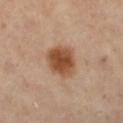{
  "patient": {
    "sex": "female",
    "age_approx": 60
  },
  "image": {
    "source": "total-body photography crop",
    "field_of_view_mm": 15
  },
  "lesion_size": {
    "long_diameter_mm_approx": 4.5
  },
  "site": "right leg",
  "automated_metrics": {
    "area_mm2_approx": 12.0,
    "cielab_L": 42,
    "cielab_a": 19,
    "cielab_b": 29,
    "vs_skin_darker_L": 12.0,
    "vs_skin_contrast_norm": 10.0
  },
  "lighting": "cross-polarized"
}